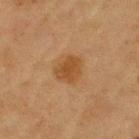Notes:
– biopsy status: total-body-photography surveillance lesion; no biopsy
– automated lesion analysis: a mean CIELAB color near L≈42 a*≈19 b*≈35 and about 8 CIELAB-L* units darker than the surrounding skin; a border-irregularity rating of about 2.5/10, a color-variation rating of about 2/10, and radial color variation of about 0.5
– patient: female, aged 78–82
– imaging modality: ~15 mm crop, total-body skin-cancer survey
– diameter: about 3.5 mm
– location: the left upper arm